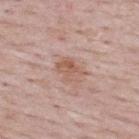Part of a total-body skin-imaging series; this lesion was reviewed on a skin check and was not flagged for biopsy. A female patient, about 50 years old. A close-up tile cropped from a whole-body skin photograph, about 15 mm across. From the upper back.